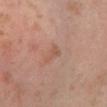Background:
The patient is a male approximately 30 years of age. About 3 mm across. The lesion is on the left lower leg. Imaged with cross-polarized lighting. A 15 mm close-up tile from a total-body photography series done for melanoma screening.